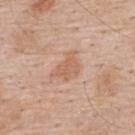Clinical impression:
The lesion was tiled from a total-body skin photograph and was not biopsied.
Context:
A male patient, aged 58 to 62. An algorithmic analysis of the crop reported an average lesion color of about L≈61 a*≈21 b*≈33 (CIELAB), roughly 7 lightness units darker than nearby skin, and a normalized border contrast of about 5.5. It also reported a detector confidence of about 100 out of 100 that the crop contains a lesion. The lesion is on the upper back. Captured under white-light illumination. A 15 mm close-up tile from a total-body photography series done for melanoma screening. The recorded lesion diameter is about 3.5 mm.